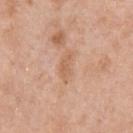The lesion was photographed on a routine skin check and not biopsied; there is no pathology result.
Approximately 3.5 mm at its widest.
Cropped from a whole-body photographic skin survey; the tile spans about 15 mm.
From the chest.
A male patient aged 48 to 52.
The tile uses white-light illumination.
The total-body-photography lesion software estimated a lesion area of about 4.5 mm² and an eccentricity of roughly 0.9. And it measured a mean CIELAB color near L≈62 a*≈21 b*≈34 and roughly 7 lightness units darker than nearby skin. It also reported border irregularity of about 3.5 on a 0–10 scale. It also reported an automated nevus-likeness rating near 0 out of 100 and a detector confidence of about 100 out of 100 that the crop contains a lesion.